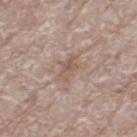About 4 mm across. The lesion-visualizer software estimated a lesion area of about 5.5 mm² and an outline eccentricity of about 0.9 (0 = round, 1 = elongated). The software also gave a lesion–skin lightness drop of about 7 and a normalized border contrast of about 5.5. And it measured border irregularity of about 4 on a 0–10 scale, internal color variation of about 3.5 on a 0–10 scale, and radial color variation of about 1.5. A female subject aged approximately 75. Captured under white-light illumination. The lesion is on the left thigh. A 15 mm crop from a total-body photograph taken for skin-cancer surveillance.